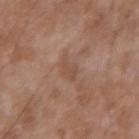Recorded during total-body skin imaging; not selected for excision or biopsy. A 15 mm crop from a total-body photograph taken for skin-cancer surveillance. Located on the left forearm. The patient is a male aged approximately 55.